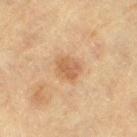follow-up = catalogued during a skin exam; not biopsied
patient = female, approximately 80 years of age
lesion size = ~3 mm (longest diameter)
TBP lesion metrics = a footprint of about 5.5 mm², a shape eccentricity near 0.6, and two-axis asymmetry of about 0.25; a mean CIELAB color near L≈51 a*≈18 b*≈33, roughly 9 lightness units darker than nearby skin, and a normalized border contrast of about 6.5; lesion-presence confidence of about 100/100
body site = the left thigh
image source = ~15 mm tile from a whole-body skin photo
illumination = cross-polarized illumination